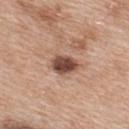Q: Was a biopsy performed?
A: total-body-photography surveillance lesion; no biopsy
Q: Automated lesion metrics?
A: a footprint of about 6.5 mm², a shape eccentricity near 0.75, and a shape-asymmetry score of about 0.15 (0 = symmetric)
Q: Who is the patient?
A: female, roughly 40 years of age
Q: What is the lesion's diameter?
A: ~3.5 mm (longest diameter)
Q: What is the imaging modality?
A: total-body-photography crop, ~15 mm field of view
Q: How was the tile lit?
A: white-light
Q: Lesion location?
A: the back